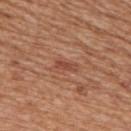Clinical impression:
This lesion was catalogued during total-body skin photography and was not selected for biopsy.
Context:
An algorithmic analysis of the crop reported an area of roughly 2.5 mm², an eccentricity of roughly 0.9, and two-axis asymmetry of about 0.25. The analysis additionally found a lesion color around L≈47 a*≈26 b*≈30 in CIELAB and a normalized lesion–skin contrast near 6.5. A male patient, in their mid- to late 60s. This is a white-light tile. A close-up tile cropped from a whole-body skin photograph, about 15 mm across. The lesion is on the upper back.